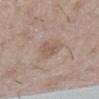body site=the right lower leg; subject=female, in their 60s; image=15 mm crop, total-body photography.